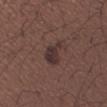Clinical impression: The lesion was tiled from a total-body skin photograph and was not biopsied. Acquisition and patient details: Captured under white-light illumination. The subject is a male aged around 40. An algorithmic analysis of the crop reported an area of roughly 6 mm² and a shape-asymmetry score of about 0.35 (0 = symmetric). The software also gave a lesion color around L≈32 a*≈16 b*≈16 in CIELAB, about 8 CIELAB-L* units darker than the surrounding skin, and a normalized border contrast of about 9. It also reported a border-irregularity index near 3.5/10, internal color variation of about 3 on a 0–10 scale, and radial color variation of about 1. And it measured a nevus-likeness score of about 40/100 and a lesion-detection confidence of about 100/100. On the left forearm. The recorded lesion diameter is about 3.5 mm. Cropped from a whole-body photographic skin survey; the tile spans about 15 mm.